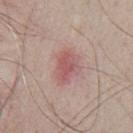• notes: imaged on a skin check; not biopsied
• TBP lesion metrics: an eccentricity of roughly 0.75 and a shape-asymmetry score of about 0.2 (0 = symmetric); a nevus-likeness score of about 10/100 and a lesion-detection confidence of about 100/100
• size: ≈3.5 mm
• subject: male, in their 50s
• imaging modality: ~15 mm crop, total-body skin-cancer survey
• site: the front of the torso